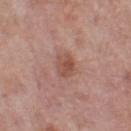Case summary:
– subject — female, roughly 50 years of age
– site — the left thigh
– lesion size — about 3 mm
– imaging modality — ~15 mm crop, total-body skin-cancer survey